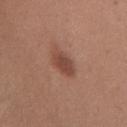  biopsy_status: not biopsied; imaged during a skin examination
  lighting: white-light
  site: chest
  image:
    source: total-body photography crop
    field_of_view_mm: 15
  patient:
    sex: female
    age_approx: 50
  automated_metrics:
    area_mm2_approx: 6.0
    eccentricity: 0.85
    shape_asymmetry: 0.2
    cielab_L: 45
    cielab_a: 22
    cielab_b: 27
    vs_skin_contrast_norm: 7.5
    border_irregularity_0_10: 2.5
    color_variation_0_10: 2.0
    peripheral_color_asymmetry: 0.5
    nevus_likeness_0_100: 85
    lesion_detection_confidence_0_100: 100
  lesion_size:
    long_diameter_mm_approx: 4.0